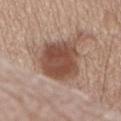Findings:
• biopsy status: catalogued during a skin exam; not biopsied
• automated lesion analysis: an average lesion color of about L≈48 a*≈20 b*≈27 (CIELAB), roughly 13 lightness units darker than nearby skin, and a normalized lesion–skin contrast near 9.5; a classifier nevus-likeness of about 95/100
• image: total-body-photography crop, ~15 mm field of view
• lighting: white-light illumination
• site: the chest
• subject: male, about 70 years old